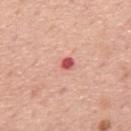follow-up = imaged on a skin check; not biopsied
patient = male, in their mid- to late 70s
imaging modality = 15 mm crop, total-body photography
lesion size = about 3 mm
anatomic site = the mid back
lighting = white-light illumination